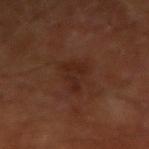site = the right forearm; acquisition = total-body-photography crop, ~15 mm field of view; illumination = cross-polarized; patient = male, aged around 65.